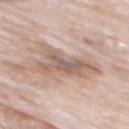Imaged with white-light lighting.
The recorded lesion diameter is about 6 mm.
Cropped from a whole-body photographic skin survey; the tile spans about 15 mm.
A male subject about 80 years old.
From the mid back.